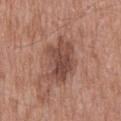Approximately 6 mm at its widest. A close-up tile cropped from a whole-body skin photograph, about 15 mm across. An algorithmic analysis of the crop reported a shape eccentricity near 0.8 and a symmetry-axis asymmetry near 0.3. The software also gave about 11 CIELAB-L* units darker than the surrounding skin and a normalized border contrast of about 8. The analysis additionally found a border-irregularity index near 4.5/10, a within-lesion color-variation index near 4.5/10, and peripheral color asymmetry of about 1.5. The analysis additionally found a nevus-likeness score of about 15/100 and a detector confidence of about 100 out of 100 that the crop contains a lesion. Captured under white-light illumination. A male patient, aged around 50. From the back.